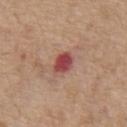| key | value |
|---|---|
| workup | no biopsy performed (imaged during a skin exam) |
| site | the chest |
| lesion size | about 3 mm |
| patient | male, roughly 70 years of age |
| image-analysis metrics | a footprint of about 5 mm², a shape eccentricity near 0.65, and a symmetry-axis asymmetry near 0.25; radial color variation of about 1 |
| image | ~15 mm tile from a whole-body skin photo |